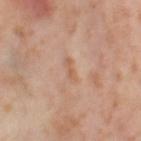Recorded during total-body skin imaging; not selected for excision or biopsy.
A lesion tile, about 15 mm wide, cut from a 3D total-body photograph.
Captured under cross-polarized illumination.
The patient is a female in their mid-50s.
The total-body-photography lesion software estimated a lesion area of about 2.5 mm², an outline eccentricity of about 0.9 (0 = round, 1 = elongated), and two-axis asymmetry of about 0.25. And it measured internal color variation of about 0 on a 0–10 scale and radial color variation of about 0. The software also gave a classifier nevus-likeness of about 0/100 and lesion-presence confidence of about 100/100.
Located on the leg.
Approximately 2.5 mm at its widest.